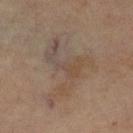follow-up — no biopsy performed (imaged during a skin exam).